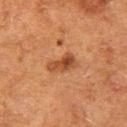• notes: total-body-photography surveillance lesion; no biopsy
• image-analysis metrics: a lesion color around L≈39 a*≈23 b*≈32 in CIELAB, a lesion–skin lightness drop of about 10, and a normalized border contrast of about 8; a border-irregularity index near 3.5/10, internal color variation of about 5 on a 0–10 scale, and a peripheral color-asymmetry measure near 1.5
• patient: female, approximately 50 years of age
• lesion size: ~3.5 mm (longest diameter)
• image source: total-body-photography crop, ~15 mm field of view
• site: the leg
• illumination: cross-polarized illumination